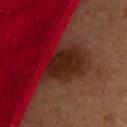Clinical impression:
Captured during whole-body skin photography for melanoma surveillance; the lesion was not biopsied.